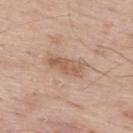No biopsy was performed on this lesion — it was imaged during a full skin examination and was not determined to be concerning.
Cropped from a total-body skin-imaging series; the visible field is about 15 mm.
A male patient roughly 65 years of age.
About 4.5 mm across.
The total-body-photography lesion software estimated a lesion area of about 7.5 mm² and an eccentricity of roughly 0.9. The software also gave an average lesion color of about L≈58 a*≈20 b*≈31 (CIELAB) and roughly 9 lightness units darker than nearby skin. The analysis additionally found a border-irregularity rating of about 2.5/10 and a peripheral color-asymmetry measure near 1.
Imaged with white-light lighting.
Located on the upper back.